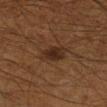<tbp_lesion>
  <biopsy_status>not biopsied; imaged during a skin examination</biopsy_status>
  <patient>
    <sex>male</sex>
    <age_approx>60</age_approx>
  </patient>
  <lighting>cross-polarized</lighting>
  <site>right lower leg</site>
  <image>
    <source>total-body photography crop</source>
    <field_of_view_mm>15</field_of_view_mm>
  </image>
  <automated_metrics>
    <area_mm2_approx>7.0</area_mm2_approx>
    <eccentricity>0.75</eccentricity>
    <shape_asymmetry>0.2</shape_asymmetry>
    <border_irregularity_0_10>2.5</border_irregularity_0_10>
    <color_variation_0_10>2.5</color_variation_0_10>
    <lesion_detection_confidence_0_100>100</lesion_detection_confidence_0_100>
  </automated_metrics>
</tbp_lesion>